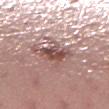From the right lower leg.
Cropped from a whole-body photographic skin survey; the tile spans about 15 mm.
The subject is a female about 55 years old.